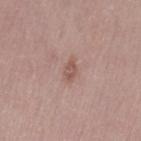biopsy status: total-body-photography surveillance lesion; no biopsy
patient: female, about 50 years old
automated lesion analysis: a footprint of about 3.5 mm² and a symmetry-axis asymmetry near 0.3; an average lesion color of about L≈54 a*≈19 b*≈25 (CIELAB) and roughly 8 lightness units darker than nearby skin
anatomic site: the left thigh
image source: 15 mm crop, total-body photography
lighting: white-light illumination